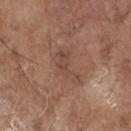  automated_metrics:
    cielab_L: 46
    cielab_a: 19
    cielab_b: 25
    border_irregularity_0_10: 6.5
    peripheral_color_asymmetry: 1.0
    lesion_detection_confidence_0_100: 100
  site: chest
  image:
    source: total-body photography crop
    field_of_view_mm: 15
  lesion_size:
    long_diameter_mm_approx: 4.5
  patient:
    sex: male
    age_approx: 70
  lighting: white-light